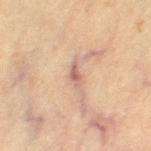Imaged during a routine full-body skin examination; the lesion was not biopsied and no histopathology is available.
A female patient, approximately 65 years of age.
From the left thigh.
The lesion-visualizer software estimated border irregularity of about 3.5 on a 0–10 scale, a color-variation rating of about 2.5/10, and radial color variation of about 0.5.
Longest diameter approximately 3 mm.
A region of skin cropped from a whole-body photographic capture, roughly 15 mm wide.